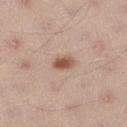Q: Was this lesion biopsied?
A: total-body-photography surveillance lesion; no biopsy
Q: How was the tile lit?
A: white-light
Q: What is the lesion's diameter?
A: ~2.5 mm (longest diameter)
Q: What are the patient's age and sex?
A: male, aged 33 to 37
Q: What did automated image analysis measure?
A: a lesion area of about 4 mm², a shape eccentricity near 0.75, and a symmetry-axis asymmetry near 0.2; border irregularity of about 2 on a 0–10 scale
Q: Lesion location?
A: the leg
Q: What kind of image is this?
A: 15 mm crop, total-body photography